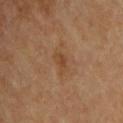Findings:
• biopsy status — total-body-photography surveillance lesion; no biopsy
• acquisition — ~15 mm tile from a whole-body skin photo
• body site — the chest
• patient — male, aged 58 to 62